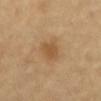Impression: Captured during whole-body skin photography for melanoma surveillance; the lesion was not biopsied. Context: About 3 mm across. This is a cross-polarized tile. A male subject, aged around 65. A 15 mm close-up tile from a total-body photography series done for melanoma screening. An algorithmic analysis of the crop reported a shape eccentricity near 0.55 and a shape-asymmetry score of about 0.2 (0 = symmetric). And it measured a border-irregularity index near 2/10, a within-lesion color-variation index near 1.5/10, and peripheral color asymmetry of about 0.5. The lesion is on the back.